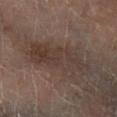Q: What are the patient's age and sex?
A: male, about 70 years old
Q: How was this image acquired?
A: 15 mm crop, total-body photography
Q: Automated lesion metrics?
A: a footprint of about 21 mm², a shape eccentricity near 0.95, and a symmetry-axis asymmetry near 0.25; a lesion color around L≈35 a*≈13 b*≈20 in CIELAB, roughly 7 lightness units darker than nearby skin, and a normalized lesion–skin contrast near 6.5
Q: Lesion size?
A: ~8.5 mm (longest diameter)
Q: Where on the body is the lesion?
A: the right lower leg
Q: Illumination type?
A: cross-polarized illumination